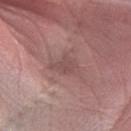follow-up: no biopsy performed (imaged during a skin exam)
acquisition: 15 mm crop, total-body photography
anatomic site: the left forearm
patient: male, approximately 75 years of age